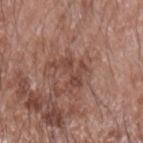<record>
  <biopsy_status>not biopsied; imaged during a skin examination</biopsy_status>
  <image>
    <source>total-body photography crop</source>
    <field_of_view_mm>15</field_of_view_mm>
  </image>
  <patient>
    <sex>male</sex>
    <age_approx>60</age_approx>
  </patient>
  <site>left forearm</site>
</record>